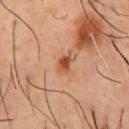Context:
A lesion tile, about 15 mm wide, cut from a 3D total-body photograph. The patient is a male aged around 55. From the front of the torso.